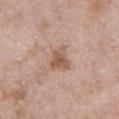Assessment: Imaged during a routine full-body skin examination; the lesion was not biopsied and no histopathology is available. Context: On the chest. The total-body-photography lesion software estimated a footprint of about 6.5 mm², a shape eccentricity near 0.65, and two-axis asymmetry of about 0.35. And it measured a lesion color around L≈56 a*≈19 b*≈29 in CIELAB, roughly 10 lightness units darker than nearby skin, and a normalized border contrast of about 7.5. The software also gave a within-lesion color-variation index near 3.5/10. It also reported a classifier nevus-likeness of about 10/100 and a lesion-detection confidence of about 100/100. A male subject, in their 50s. A lesion tile, about 15 mm wide, cut from a 3D total-body photograph. This is a white-light tile. The recorded lesion diameter is about 3 mm.